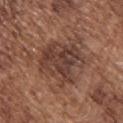About 6 mm across. Located on the upper back. A male patient roughly 75 years of age. A roughly 15 mm field-of-view crop from a total-body skin photograph.